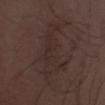subject=male, aged around 30
imaging modality=total-body-photography crop, ~15 mm field of view
location=the left lower leg